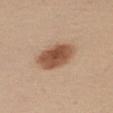  biopsy_status: not biopsied; imaged during a skin examination
  patient:
    sex: male
    age_approx: 50
  image:
    source: total-body photography crop
    field_of_view_mm: 15
  site: left arm